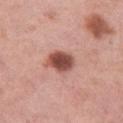Case summary:
– workup: no biopsy performed (imaged during a skin exam)
– anatomic site: the left thigh
– acquisition: total-body-photography crop, ~15 mm field of view
– lesion diameter: about 3.5 mm
– image-analysis metrics: a border-irregularity index near 1.5/10, internal color variation of about 4.5 on a 0–10 scale, and a peripheral color-asymmetry measure near 1
– subject: female, about 50 years old